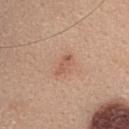The lesion was photographed on a routine skin check and not biopsied; there is no pathology result.
On the upper back.
About 3 mm across.
This is a white-light tile.
A female patient in their mid- to late 30s.
The total-body-photography lesion software estimated a within-lesion color-variation index near 0/10 and radial color variation of about 0. And it measured a classifier nevus-likeness of about 30/100 and lesion-presence confidence of about 100/100.
A region of skin cropped from a whole-body photographic capture, roughly 15 mm wide.